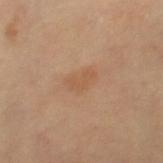biopsy status — total-body-photography surveillance lesion; no biopsy | image — ~15 mm tile from a whole-body skin photo | patient — female, approximately 60 years of age | illumination — cross-polarized illumination | location — the left leg | lesion size — about 3.5 mm.